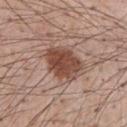Impression:
Part of a total-body skin-imaging series; this lesion was reviewed on a skin check and was not flagged for biopsy.
Acquisition and patient details:
A 15 mm close-up tile from a total-body photography series done for melanoma screening. The lesion is on the chest. Imaged with white-light lighting. The subject is a male aged approximately 55. Automated tile analysis of the lesion measured an area of roughly 15 mm², a shape eccentricity near 0.7, and two-axis asymmetry of about 0.15. It also reported border irregularity of about 2 on a 0–10 scale and a color-variation rating of about 4.5/10.